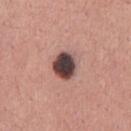follow-up — total-body-photography surveillance lesion; no biopsy | image — total-body-photography crop, ~15 mm field of view | illumination — white-light illumination | anatomic site — the front of the torso | subject — male, in their mid- to late 40s | TBP lesion metrics — a lesion area of about 8.5 mm², an eccentricity of roughly 0.5, and two-axis asymmetry of about 0.1.